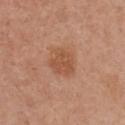Q: Is there a histopathology result?
A: imaged on a skin check; not biopsied
Q: What lighting was used for the tile?
A: white-light illumination
Q: Who is the patient?
A: female, approximately 40 years of age
Q: Automated lesion metrics?
A: an average lesion color of about L≈52 a*≈24 b*≈33 (CIELAB), about 8 CIELAB-L* units darker than the surrounding skin, and a lesion-to-skin contrast of about 6.5 (normalized; higher = more distinct); radial color variation of about 1; an automated nevus-likeness rating near 30 out of 100
Q: What is the lesion's diameter?
A: about 4 mm
Q: What is the imaging modality?
A: total-body-photography crop, ~15 mm field of view
Q: Lesion location?
A: the front of the torso